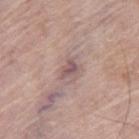{"biopsy_status": "not biopsied; imaged during a skin examination", "image": {"source": "total-body photography crop", "field_of_view_mm": 15}, "automated_metrics": {"area_mm2_approx": 3.5, "eccentricity": 0.75, "shape_asymmetry": 0.4, "cielab_L": 54, "cielab_a": 18, "cielab_b": 18, "vs_skin_contrast_norm": 7.5, "nevus_likeness_0_100": 0}, "site": "right thigh", "patient": {"sex": "female", "age_approx": 75}, "lighting": "white-light", "lesion_size": {"long_diameter_mm_approx": 2.5}}